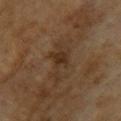biopsy status — catalogued during a skin exam; not biopsied
imaging modality — ~15 mm tile from a whole-body skin photo
subject — female, in their 60s
location — the back